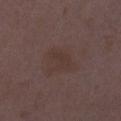A 15 mm crop from a total-body photograph taken for skin-cancer surveillance. Captured under white-light illumination. A female patient aged around 35. The lesion is on the left thigh. About 2.5 mm across.